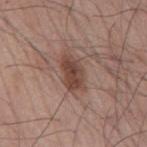Imaged during a routine full-body skin examination; the lesion was not biopsied and no histopathology is available.